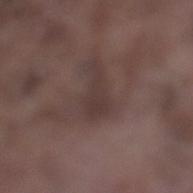Assessment:
No biopsy was performed on this lesion — it was imaged during a full skin examination and was not determined to be concerning.
Background:
The patient is a male roughly 75 years of age. On the left lower leg. Imaged with white-light lighting. A 15 mm close-up tile from a total-body photography series done for melanoma screening. Longest diameter approximately 4 mm.